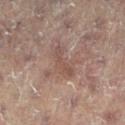Part of a total-body skin-imaging series; this lesion was reviewed on a skin check and was not flagged for biopsy. The lesion's longest dimension is about 4 mm. A female patient approximately 80 years of age. A lesion tile, about 15 mm wide, cut from a 3D total-body photograph. Imaged with cross-polarized lighting. On the right leg.